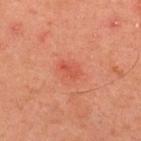- notes: catalogued during a skin exam; not biopsied
- image source: ~15 mm crop, total-body skin-cancer survey
- location: the upper back
- subject: male, in their mid- to late 40s
- TBP lesion metrics: an average lesion color of about L≈44 a*≈30 b*≈30 (CIELAB), a lesion–skin lightness drop of about 6, and a lesion-to-skin contrast of about 4.5 (normalized; higher = more distinct); a border-irregularity rating of about 3/10 and internal color variation of about 2.5 on a 0–10 scale; an automated nevus-likeness rating near 0 out of 100 and a detector confidence of about 100 out of 100 that the crop contains a lesion